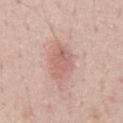follow-up — total-body-photography surveillance lesion; no biopsy | imaging modality — ~15 mm crop, total-body skin-cancer survey | site — the front of the torso | lesion size — ~5 mm (longest diameter) | subject — male, about 70 years old | automated metrics — an automated nevus-likeness rating near 30 out of 100 | tile lighting — white-light.